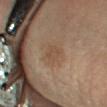Recorded during total-body skin imaging; not selected for excision or biopsy. A female patient, aged 58 to 62. Located on the right forearm. Cropped from a whole-body photographic skin survey; the tile spans about 15 mm.